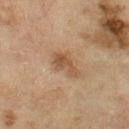Clinical summary:
A female patient, about 55 years old. Measured at roughly 4 mm in maximum diameter. A 15 mm close-up tile from a total-body photography series done for melanoma screening. Located on the left thigh. Automated image analysis of the tile measured a lesion color around L≈45 a*≈16 b*≈30 in CIELAB and a lesion-to-skin contrast of about 6.5 (normalized; higher = more distinct). It also reported a border-irregularity rating of about 4.5/10 and peripheral color asymmetry of about 1. The software also gave a nevus-likeness score of about 10/100 and a lesion-detection confidence of about 100/100.